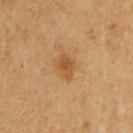Background:
About 2.5 mm across. The patient is a male roughly 75 years of age. A region of skin cropped from a whole-body photographic capture, roughly 15 mm wide. The total-body-photography lesion software estimated an area of roughly 4 mm², an eccentricity of roughly 0.75, and two-axis asymmetry of about 0.15. The software also gave border irregularity of about 1.5 on a 0–10 scale and peripheral color asymmetry of about 0.5. From the chest. This is a cross-polarized tile.